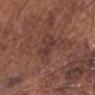Recorded during total-body skin imaging; not selected for excision or biopsy.
Measured at roughly 3 mm in maximum diameter.
A region of skin cropped from a whole-body photographic capture, roughly 15 mm wide.
The lesion is located on the head or neck.
The patient is a male aged approximately 75.